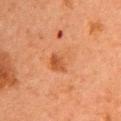Case summary:
– workup — catalogued during a skin exam; not biopsied
– TBP lesion metrics — roughly 6 lightness units darker than nearby skin and a normalized lesion–skin contrast near 5; an automated nevus-likeness rating near 0 out of 100 and lesion-presence confidence of about 100/100
– illumination — cross-polarized
– image — total-body-photography crop, ~15 mm field of view
– subject — female, approximately 60 years of age
– location — the left upper arm